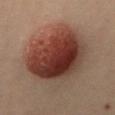This lesion was catalogued during total-body skin photography and was not selected for biopsy. Captured under cross-polarized illumination. Cropped from a total-body skin-imaging series; the visible field is about 15 mm. The patient is a female aged 28–32. Approximately 8.5 mm at its widest. The lesion-visualizer software estimated a lesion color around L≈29 a*≈18 b*≈21 in CIELAB and a lesion–skin lightness drop of about 12. The software also gave border irregularity of about 2 on a 0–10 scale, a within-lesion color-variation index near 8.5/10, and a peripheral color-asymmetry measure near 2.5. The software also gave an automated nevus-likeness rating near 100 out of 100 and lesion-presence confidence of about 100/100. The lesion is on the front of the torso.